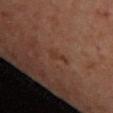Clinical impression: Imaged during a routine full-body skin examination; the lesion was not biopsied and no histopathology is available. Acquisition and patient details: A region of skin cropped from a whole-body photographic capture, roughly 15 mm wide. A female patient, in their mid- to late 40s. The lesion-visualizer software estimated an area of roughly 2.5 mm² and a shape-asymmetry score of about 0.4 (0 = symmetric). The analysis additionally found a normalized lesion–skin contrast near 5.5. And it measured an automated nevus-likeness rating near 0 out of 100. The recorded lesion diameter is about 3 mm. On the upper back.